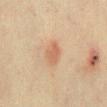Assessment: The lesion was photographed on a routine skin check and not biopsied; there is no pathology result. Acquisition and patient details: Cropped from a total-body skin-imaging series; the visible field is about 15 mm. Captured under cross-polarized illumination. Automated image analysis of the tile measured an area of roughly 4 mm² and an eccentricity of roughly 0.7. The software also gave a mean CIELAB color near L≈52 a*≈19 b*≈30, about 8 CIELAB-L* units darker than the surrounding skin, and a lesion-to-skin contrast of about 6 (normalized; higher = more distinct). The software also gave border irregularity of about 2 on a 0–10 scale and radial color variation of about 0.5. The analysis additionally found a nevus-likeness score of about 60/100 and a detector confidence of about 100 out of 100 that the crop contains a lesion. From the chest. A male patient, roughly 75 years of age.